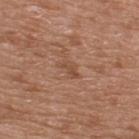Q: Was this lesion biopsied?
A: total-body-photography surveillance lesion; no biopsy
Q: Where on the body is the lesion?
A: the upper back
Q: How large is the lesion?
A: ≈2.5 mm
Q: What are the patient's age and sex?
A: male, aged 48–52
Q: How was this image acquired?
A: ~15 mm tile from a whole-body skin photo